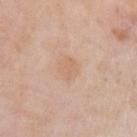This lesion was catalogued during total-body skin photography and was not selected for biopsy. The lesion-visualizer software estimated a footprint of about 4 mm², an eccentricity of roughly 0.75, and a symmetry-axis asymmetry near 0.35. The software also gave a border-irregularity rating of about 3.5/10, a color-variation rating of about 2/10, and peripheral color asymmetry of about 0.5. A 15 mm crop from a total-body photograph taken for skin-cancer surveillance. Located on the left upper arm. Imaged with white-light lighting. Measured at roughly 3 mm in maximum diameter. A female subject aged approximately 60.